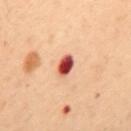The lesion was photographed on a routine skin check and not biopsied; there is no pathology result. The lesion's longest dimension is about 2.5 mm. The patient is a female about 50 years old. On the back. Cropped from a whole-body photographic skin survey; the tile spans about 15 mm.